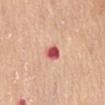Q: Was this lesion biopsied?
A: imaged on a skin check; not biopsied
Q: Patient demographics?
A: female, aged around 65
Q: How was the tile lit?
A: white-light
Q: Lesion location?
A: the front of the torso
Q: What is the imaging modality?
A: 15 mm crop, total-body photography
Q: What is the lesion's diameter?
A: ~2.5 mm (longest diameter)
Q: Automated lesion metrics?
A: a mean CIELAB color near L≈56 a*≈37 b*≈27 and a lesion–skin lightness drop of about 18; an automated nevus-likeness rating near 0 out of 100 and a detector confidence of about 100 out of 100 that the crop contains a lesion